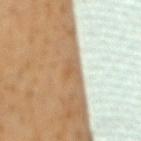  biopsy_status: not biopsied; imaged during a skin examination
  automated_metrics:
    cielab_L: 53
    cielab_a: 15
    cielab_b: 32
    vs_skin_contrast_norm: 7.0
    border_irregularity_0_10: 3.5
    color_variation_0_10: 2.0
    peripheral_color_asymmetry: 0.5
    nevus_likeness_0_100: 0
    lesion_detection_confidence_0_100: 75
  patient:
    sex: male
    age_approx: 60
  site: mid back
  lighting: cross-polarized
  image:
    source: total-body photography crop
    field_of_view_mm: 15